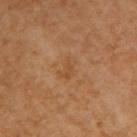Clinical impression: Captured during whole-body skin photography for melanoma surveillance; the lesion was not biopsied. Image and clinical context: An algorithmic analysis of the crop reported an outline eccentricity of about 0.8 (0 = round, 1 = elongated) and two-axis asymmetry of about 0.35. The analysis additionally found lesion-presence confidence of about 100/100. A female patient aged 48 to 52. The lesion is located on the arm. Imaged with cross-polarized lighting. Approximately 3 mm at its widest. A 15 mm crop from a total-body photograph taken for skin-cancer surveillance.